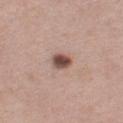Clinical impression:
No biopsy was performed on this lesion — it was imaged during a full skin examination and was not determined to be concerning.